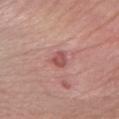notes: catalogued during a skin exam; not biopsied | location: the right forearm | acquisition: 15 mm crop, total-body photography | lesion diameter: ~3 mm (longest diameter) | subject: female, roughly 55 years of age.